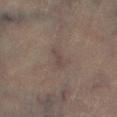Clinical impression: The lesion was tiled from a total-body skin photograph and was not biopsied. Clinical summary: A male patient, aged 63 to 67. This is a cross-polarized tile. The recorded lesion diameter is about 3 mm. The lesion is located on the right lower leg. A 15 mm close-up tile from a total-body photography series done for melanoma screening.